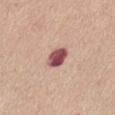The lesion was photographed on a routine skin check and not biopsied; there is no pathology result.
The tile uses white-light illumination.
The recorded lesion diameter is about 3 mm.
Automated tile analysis of the lesion measured an outline eccentricity of about 0.75 (0 = round, 1 = elongated) and a shape-asymmetry score of about 0.2 (0 = symmetric). It also reported a classifier nevus-likeness of about 0/100 and lesion-presence confidence of about 100/100.
A female patient, aged approximately 55.
Located on the abdomen.
A roughly 15 mm field-of-view crop from a total-body skin photograph.